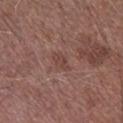{
  "biopsy_status": "not biopsied; imaged during a skin examination",
  "patient": {
    "sex": "male",
    "age_approx": 75
  },
  "image": {
    "source": "total-body photography crop",
    "field_of_view_mm": 15
  },
  "site": "leg"
}